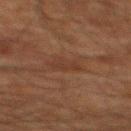size=~3 mm (longest diameter)
image source=~15 mm tile from a whole-body skin photo
location=the mid back
subject=male, aged around 60
tile lighting=cross-polarized